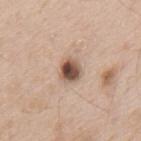The lesion is on the back. Cropped from a total-body skin-imaging series; the visible field is about 15 mm. A male patient, aged around 65.